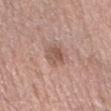Case summary:
- follow-up: catalogued during a skin exam; not biopsied
- image-analysis metrics: a lesion area of about 6 mm², an outline eccentricity of about 0.65 (0 = round, 1 = elongated), and a symmetry-axis asymmetry near 0.25; a mean CIELAB color near L≈55 a*≈20 b*≈25, about 10 CIELAB-L* units darker than the surrounding skin, and a lesion-to-skin contrast of about 6.5 (normalized; higher = more distinct); a border-irregularity rating of about 2.5/10, a color-variation rating of about 4/10, and peripheral color asymmetry of about 1.5
- lighting: white-light illumination
- acquisition: ~15 mm tile from a whole-body skin photo
- site: the right forearm
- size: ≈3 mm
- patient: male, approximately 65 years of age